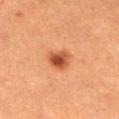Captured during whole-body skin photography for melanoma surveillance; the lesion was not biopsied.
The patient is a female aged around 55.
Automated image analysis of the tile measured an area of roughly 6.5 mm², an eccentricity of roughly 0.65, and a symmetry-axis asymmetry near 0.2. The software also gave a lesion-detection confidence of about 100/100.
A lesion tile, about 15 mm wide, cut from a 3D total-body photograph.
Located on the left lower leg.